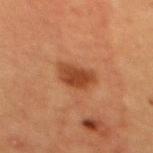<case>
<biopsy_status>not biopsied; imaged during a skin examination</biopsy_status>
<image>
  <source>total-body photography crop</source>
  <field_of_view_mm>15</field_of_view_mm>
</image>
<patient>
  <sex>female</sex>
  <age_approx>40</age_approx>
</patient>
<lighting>cross-polarized</lighting>
<site>mid back</site>
</case>